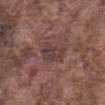  biopsy_status: not biopsied; imaged during a skin examination
  site: abdomen
  lesion_size:
    long_diameter_mm_approx: 3.5
  patient:
    sex: male
    age_approx: 75
  image:
    source: total-body photography crop
    field_of_view_mm: 15